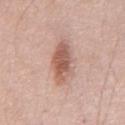tile lighting: white-light illumination | anatomic site: the chest | patient: male, roughly 55 years of age | size: ~6 mm (longest diameter) | imaging modality: ~15 mm crop, total-body skin-cancer survey | image-analysis metrics: a mean CIELAB color near L≈58 a*≈22 b*≈27, about 13 CIELAB-L* units darker than the surrounding skin, and a lesion-to-skin contrast of about 8 (normalized; higher = more distinct); a border-irregularity rating of about 2.5/10 and a color-variation rating of about 4.5/10.